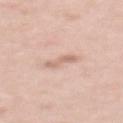Imaged during a routine full-body skin examination; the lesion was not biopsied and no histopathology is available. Cropped from a whole-body photographic skin survey; the tile spans about 15 mm. This is a white-light tile. From the upper back. A female patient, aged around 40.